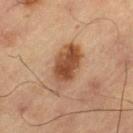notes — imaged on a skin check; not biopsied | image — ~15 mm crop, total-body skin-cancer survey | subject — male, aged 63–67 | site — the right thigh.